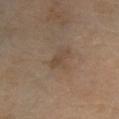workup = imaged on a skin check; not biopsied | diameter = ≈3 mm | acquisition = ~15 mm tile from a whole-body skin photo | tile lighting = cross-polarized | site = the right lower leg | subject = female, roughly 70 years of age.